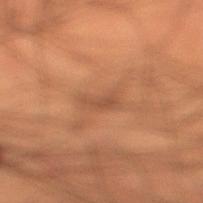Q: Was a biopsy performed?
A: total-body-photography surveillance lesion; no biopsy